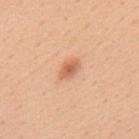Imaged during a routine full-body skin examination; the lesion was not biopsied and no histopathology is available. Cropped from a whole-body photographic skin survey; the tile spans about 15 mm. The lesion-visualizer software estimated an area of roughly 4 mm², an eccentricity of roughly 0.8, and two-axis asymmetry of about 0.3. The software also gave a lesion–skin lightness drop of about 12 and a normalized lesion–skin contrast near 7. It also reported a border-irregularity index near 2.5/10, a within-lesion color-variation index near 3.5/10, and radial color variation of about 1. And it measured a classifier nevus-likeness of about 90/100 and a detector confidence of about 100 out of 100 that the crop contains a lesion. Captured under white-light illumination. Approximately 3 mm at its widest. A female patient, aged 43–47. The lesion is located on the back.